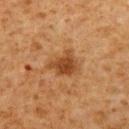follow-up: catalogued during a skin exam; not biopsied | lesion diameter: ~3.5 mm (longest diameter) | anatomic site: the left upper arm | TBP lesion metrics: a footprint of about 7 mm², a shape eccentricity near 0.5, and two-axis asymmetry of about 0.35; a mean CIELAB color near L≈38 a*≈21 b*≈34, a lesion–skin lightness drop of about 10, and a lesion-to-skin contrast of about 8.5 (normalized; higher = more distinct); a border-irregularity index near 3.5/10, internal color variation of about 2.5 on a 0–10 scale, and a peripheral color-asymmetry measure near 1; an automated nevus-likeness rating near 75 out of 100 and a detector confidence of about 100 out of 100 that the crop contains a lesion | tile lighting: cross-polarized | subject: male, aged approximately 60 | acquisition: ~15 mm tile from a whole-body skin photo.